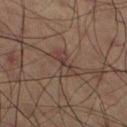Recorded during total-body skin imaging; not selected for excision or biopsy. The recorded lesion diameter is about 3.5 mm. A male subject, about 60 years old. Cropped from a whole-body photographic skin survey; the tile spans about 15 mm.